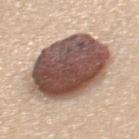workup — imaged on a skin check; not biopsied
patient — female, roughly 35 years of age
anatomic site — the back
tile lighting — white-light
image source — ~15 mm crop, total-body skin-cancer survey